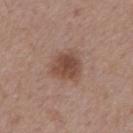biopsy_status: not biopsied; imaged during a skin examination
image:
  source: total-body photography crop
  field_of_view_mm: 15
patient:
  sex: male
  age_approx: 55